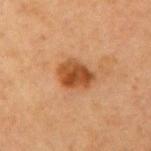Q: Was this lesion biopsied?
A: no biopsy performed (imaged during a skin exam)